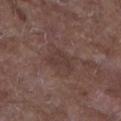notes — no biopsy performed (imaged during a skin exam) | subject — male, aged 63–67 | acquisition — ~15 mm crop, total-body skin-cancer survey | diameter — about 3 mm | illumination — white-light illumination | body site — the left forearm | TBP lesion metrics — an area of roughly 5 mm²; a lesion color around L≈36 a*≈17 b*≈20 in CIELAB and about 6 CIELAB-L* units darker than the surrounding skin; internal color variation of about 1.5 on a 0–10 scale and a peripheral color-asymmetry measure near 0.5; a nevus-likeness score of about 0/100.